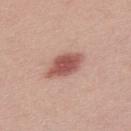Notes:
– notes — no biopsy performed (imaged during a skin exam)
– image — ~15 mm tile from a whole-body skin photo
– subject — male, roughly 30 years of age